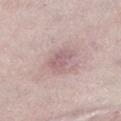Q: Is there a histopathology result?
A: imaged on a skin check; not biopsied
Q: What is the imaging modality?
A: 15 mm crop, total-body photography
Q: What are the patient's age and sex?
A: female, aged around 65
Q: What is the lesion's diameter?
A: about 3 mm
Q: Automated lesion metrics?
A: a footprint of about 6.5 mm² and a symmetry-axis asymmetry near 0.3; a lesion color around L≈60 a*≈20 b*≈17 in CIELAB, about 8 CIELAB-L* units darker than the surrounding skin, and a normalized lesion–skin contrast near 5.5; a border-irregularity rating of about 3/10 and a peripheral color-asymmetry measure near 1; a classifier nevus-likeness of about 0/100 and a detector confidence of about 85 out of 100 that the crop contains a lesion
Q: What is the anatomic site?
A: the leg